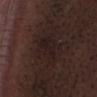{"biopsy_status": "not biopsied; imaged during a skin examination", "site": "chest", "patient": {"sex": "male", "age_approx": 70}, "image": {"source": "total-body photography crop", "field_of_view_mm": 15}, "automated_metrics": {"cielab_L": 18, "cielab_a": 12, "cielab_b": 14, "vs_skin_darker_L": 4.0, "vs_skin_contrast_norm": 6.5}}